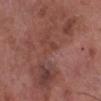Case summary:
• workup: catalogued during a skin exam; not biopsied
• patient: male, aged approximately 85 or older
• image source: total-body-photography crop, ~15 mm field of view
• diameter: ~14.5 mm (longest diameter)
• site: the left lower leg
• illumination: white-light